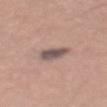biopsy status = catalogued during a skin exam; not biopsied | lesion diameter = about 4.5 mm | image source = ~15 mm tile from a whole-body skin photo | body site = the back | subject = male, in their mid- to late 40s | TBP lesion metrics = internal color variation of about 4 on a 0–10 scale and radial color variation of about 1; an automated nevus-likeness rating near 35 out of 100 and lesion-presence confidence of about 100/100 | lighting = white-light.